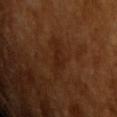The lesion was tiled from a total-body skin photograph and was not biopsied.
A male patient in their mid-60s.
This is a cross-polarized tile.
A close-up tile cropped from a whole-body skin photograph, about 15 mm across.
The total-body-photography lesion software estimated a lesion area of about 6.5 mm², an outline eccentricity of about 0.9 (0 = round, 1 = elongated), and a symmetry-axis asymmetry near 0.4. It also reported an average lesion color of about L≈22 a*≈19 b*≈27 (CIELAB), about 5 CIELAB-L* units darker than the surrounding skin, and a normalized lesion–skin contrast near 5.5. The software also gave a nevus-likeness score of about 5/100 and a lesion-detection confidence of about 100/100.